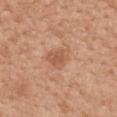Q: How large is the lesion?
A: about 3.5 mm
Q: Lesion location?
A: the mid back
Q: What lighting was used for the tile?
A: white-light illumination
Q: What are the patient's age and sex?
A: female, approximately 40 years of age
Q: What did automated image analysis measure?
A: a footprint of about 6.5 mm², a shape eccentricity near 0.7, and two-axis asymmetry of about 0.3; a mean CIELAB color near L≈57 a*≈23 b*≈34 and roughly 8 lightness units darker than nearby skin; a classifier nevus-likeness of about 15/100 and lesion-presence confidence of about 100/100
Q: What kind of image is this?
A: total-body-photography crop, ~15 mm field of view